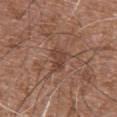notes: imaged on a skin check; not biopsied
patient: male, aged 73–77
TBP lesion metrics: an outline eccentricity of about 0.75 (0 = round, 1 = elongated); border irregularity of about 3.5 on a 0–10 scale, a within-lesion color-variation index near 3.5/10, and a peripheral color-asymmetry measure near 1
site: the abdomen
image: ~15 mm tile from a whole-body skin photo
illumination: white-light